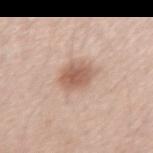{
  "biopsy_status": "not biopsied; imaged during a skin examination",
  "lighting": "white-light",
  "lesion_size": {
    "long_diameter_mm_approx": 3.0
  },
  "site": "left forearm",
  "image": {
    "source": "total-body photography crop",
    "field_of_view_mm": 15
  },
  "patient": {
    "sex": "female",
    "age_approx": 40
  }
}